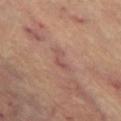Case summary:
• follow-up — imaged on a skin check; not biopsied
• subject — female, approximately 65 years of age
• site — the left thigh
• diameter — ~3 mm (longest diameter)
• tile lighting — cross-polarized illumination
• acquisition — ~15 mm crop, total-body skin-cancer survey